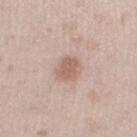The lesion is located on the left lower leg. A lesion tile, about 15 mm wide, cut from a 3D total-body photograph. A female patient, in their mid- to late 20s.